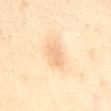Part of a total-body skin-imaging series; this lesion was reviewed on a skin check and was not flagged for biopsy.
Cropped from a whole-body photographic skin survey; the tile spans about 15 mm.
Approximately 3 mm at its widest.
Automated tile analysis of the lesion measured a footprint of about 3 mm² and an eccentricity of roughly 0.95.
From the mid back.
Captured under cross-polarized illumination.
A female subject aged approximately 35.